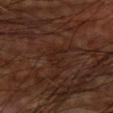{
  "site": "left upper arm",
  "patient": {
    "sex": "male",
    "age_approx": 60
  },
  "image": {
    "source": "total-body photography crop",
    "field_of_view_mm": 15
  }
}